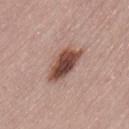The lesion was photographed on a routine skin check and not biopsied; there is no pathology result. The lesion is on the lower back. The tile uses white-light illumination. The total-body-photography lesion software estimated a lesion color around L≈47 a*≈21 b*≈25 in CIELAB, roughly 17 lightness units darker than nearby skin, and a normalized border contrast of about 12. A 15 mm crop from a total-body photograph taken for skin-cancer surveillance. The patient is a female roughly 50 years of age.